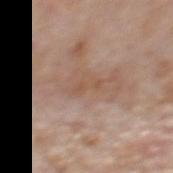Clinical impression:
Recorded during total-body skin imaging; not selected for excision or biopsy.